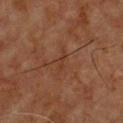Impression:
Captured during whole-body skin photography for melanoma surveillance; the lesion was not biopsied.
Acquisition and patient details:
A male patient roughly 60 years of age. A lesion tile, about 15 mm wide, cut from a 3D total-body photograph. On the front of the torso.